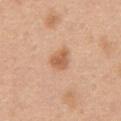Q: Is there a histopathology result?
A: catalogued during a skin exam; not biopsied
Q: What did automated image analysis measure?
A: a footprint of about 5 mm², an eccentricity of roughly 0.6, and a symmetry-axis asymmetry near 0.35; a classifier nevus-likeness of about 95/100 and lesion-presence confidence of about 100/100
Q: What is the imaging modality?
A: total-body-photography crop, ~15 mm field of view
Q: Patient demographics?
A: male, aged 48–52
Q: What is the anatomic site?
A: the chest
Q: Lesion size?
A: about 3 mm
Q: How was the tile lit?
A: white-light